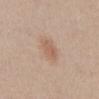Q: Was this lesion biopsied?
A: imaged on a skin check; not biopsied
Q: How large is the lesion?
A: ~3 mm (longest diameter)
Q: Who is the patient?
A: female, aged 43–47
Q: What is the anatomic site?
A: the abdomen
Q: How was the tile lit?
A: white-light
Q: What is the imaging modality?
A: ~15 mm crop, total-body skin-cancer survey